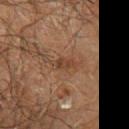Notes:
* workup · imaged on a skin check; not biopsied
* tile lighting · cross-polarized
* site · the left forearm
* image source · 15 mm crop, total-body photography
* lesion size · about 3.5 mm
* patient · male, aged around 70